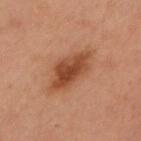This lesion was catalogued during total-body skin photography and was not selected for biopsy. The recorded lesion diameter is about 6 mm. Cropped from a total-body skin-imaging series; the visible field is about 15 mm. The subject is a female aged 53–57. Located on the chest. Imaged with cross-polarized lighting.